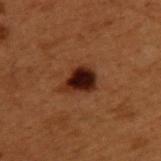The lesion was tiled from a total-body skin photograph and was not biopsied. This is a cross-polarized tile. Longest diameter approximately 4 mm. Located on the upper back. Automated image analysis of the tile measured an average lesion color of about L≈18 a*≈19 b*≈21 (CIELAB), a lesion–skin lightness drop of about 13, and a normalized lesion–skin contrast near 15. A male subject, aged approximately 50. A roughly 15 mm field-of-view crop from a total-body skin photograph.